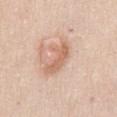follow-up = no biopsy performed (imaged during a skin exam)
lighting = white-light
lesion diameter = ≈5 mm
patient = female, aged around 45
anatomic site = the abdomen
TBP lesion metrics = an eccentricity of roughly 0.9 and two-axis asymmetry of about 0.4; an average lesion color of about L≈64 a*≈21 b*≈32 (CIELAB) and about 10 CIELAB-L* units darker than the surrounding skin
imaging modality = ~15 mm crop, total-body skin-cancer survey